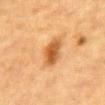Q: Is there a histopathology result?
A: imaged on a skin check; not biopsied
Q: What are the patient's age and sex?
A: male, about 85 years old or older
Q: How was this image acquired?
A: 15 mm crop, total-body photography
Q: What lighting was used for the tile?
A: cross-polarized
Q: Where on the body is the lesion?
A: the chest
Q: Lesion size?
A: ~4 mm (longest diameter)
Q: What did automated image analysis measure?
A: a lesion color around L≈51 a*≈22 b*≈40 in CIELAB and a lesion–skin lightness drop of about 12; border irregularity of about 2 on a 0–10 scale, internal color variation of about 4 on a 0–10 scale, and a peripheral color-asymmetry measure near 1; a classifier nevus-likeness of about 95/100